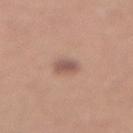<record>
<biopsy_status>not biopsied; imaged during a skin examination</biopsy_status>
<patient>
  <sex>female</sex>
  <age_approx>50</age_approx>
</patient>
<lesion_size>
  <long_diameter_mm_approx>2.5</long_diameter_mm_approx>
</lesion_size>
<image>
  <source>total-body photography crop</source>
  <field_of_view_mm>15</field_of_view_mm>
</image>
<lighting>white-light</lighting>
<automated_metrics>
  <area_mm2_approx>5.0</area_mm2_approx>
  <cielab_L>55</cielab_L>
  <cielab_a>19</cielab_a>
  <cielab_b>25</cielab_b>
  <vs_skin_contrast_norm>7.0</vs_skin_contrast_norm>
  <nevus_likeness_0_100>70</nevus_likeness_0_100>
  <lesion_detection_confidence_0_100>100</lesion_detection_confidence_0_100>
</automated_metrics>
<site>abdomen</site>
</record>